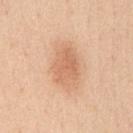Assessment:
No biopsy was performed on this lesion — it was imaged during a full skin examination and was not determined to be concerning.
Background:
The tile uses white-light illumination. On the chest. A male patient, approximately 50 years of age. Cropped from a total-body skin-imaging series; the visible field is about 15 mm.